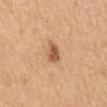follow-up — catalogued during a skin exam; not biopsied
lighting — white-light
body site — the mid back
automated lesion analysis — a footprint of about 4 mm² and an outline eccentricity of about 0.7 (0 = round, 1 = elongated); a nevus-likeness score of about 85/100
patient — female, aged around 55
size — ≈2.5 mm
image source — ~15 mm crop, total-body skin-cancer survey